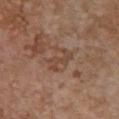This lesion was catalogued during total-body skin photography and was not selected for biopsy. Approximately 3.5 mm at its widest. A female patient, in their mid- to late 60s. Imaged with white-light lighting. Cropped from a total-body skin-imaging series; the visible field is about 15 mm. Located on the chest.